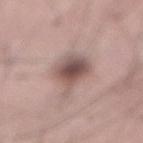Q: Was a biopsy performed?
A: imaged on a skin check; not biopsied
Q: How was the tile lit?
A: white-light illumination
Q: Where on the body is the lesion?
A: the abdomen
Q: What is the lesion's diameter?
A: ≈6.5 mm
Q: How was this image acquired?
A: 15 mm crop, total-body photography
Q: Patient demographics?
A: male, in their mid-50s